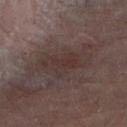This lesion was catalogued during total-body skin photography and was not selected for biopsy.
From the right leg.
Captured under cross-polarized illumination.
A 15 mm close-up tile from a total-body photography series done for melanoma screening.
About 6.5 mm across.
A female patient approximately 80 years of age.
The total-body-photography lesion software estimated an area of roughly 14 mm², an outline eccentricity of about 0.9 (0 = round, 1 = elongated), and a shape-asymmetry score of about 0.3 (0 = symmetric). The software also gave a mean CIELAB color near L≈29 a*≈12 b*≈15, about 6 CIELAB-L* units darker than the surrounding skin, and a lesion-to-skin contrast of about 6 (normalized; higher = more distinct). The software also gave a border-irregularity rating of about 4.5/10, a color-variation rating of about 3.5/10, and a peripheral color-asymmetry measure near 1. It also reported a nevus-likeness score of about 0/100 and a detector confidence of about 50 out of 100 that the crop contains a lesion.